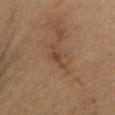Q: What is the imaging modality?
A: ~15 mm crop, total-body skin-cancer survey
Q: Patient demographics?
A: female, aged around 40
Q: How large is the lesion?
A: about 4 mm
Q: What did automated image analysis measure?
A: an average lesion color of about L≈35 a*≈15 b*≈26 (CIELAB), a lesion–skin lightness drop of about 6, and a normalized border contrast of about 6; a border-irregularity index near 5/10 and radial color variation of about 0.5
Q: Illumination type?
A: cross-polarized
Q: What is the anatomic site?
A: the right upper arm